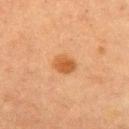Captured during whole-body skin photography for melanoma surveillance; the lesion was not biopsied.
This is a cross-polarized tile.
A lesion tile, about 15 mm wide, cut from a 3D total-body photograph.
A female patient, aged 58 to 62.
On the chest.
About 2.5 mm across.
Automated tile analysis of the lesion measured an automated nevus-likeness rating near 100 out of 100 and lesion-presence confidence of about 100/100.